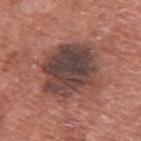Q: Was this lesion biopsied?
A: total-body-photography surveillance lesion; no biopsy
Q: What lighting was used for the tile?
A: white-light illumination
Q: How was this image acquired?
A: 15 mm crop, total-body photography
Q: What are the patient's age and sex?
A: male, approximately 75 years of age
Q: How large is the lesion?
A: about 7 mm
Q: What did automated image analysis measure?
A: a border-irregularity index near 2.5/10, a within-lesion color-variation index near 7/10, and peripheral color asymmetry of about 2; a lesion-detection confidence of about 100/100
Q: Lesion location?
A: the upper back